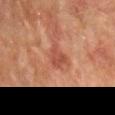{
  "biopsy_status": "not biopsied; imaged during a skin examination",
  "image": {
    "source": "total-body photography crop",
    "field_of_view_mm": 15
  },
  "lighting": "cross-polarized",
  "automated_metrics": {
    "nevus_likeness_0_100": 0,
    "lesion_detection_confidence_0_100": 100
  },
  "site": "mid back",
  "patient": {
    "sex": "male",
    "age_approx": 75
  },
  "lesion_size": {
    "long_diameter_mm_approx": 3.5
  }
}